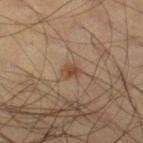{"biopsy_status": "not biopsied; imaged during a skin examination", "image": {"source": "total-body photography crop", "field_of_view_mm": 15}, "site": "leg", "patient": {"sex": "male", "age_approx": 45}}